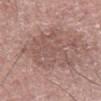Recorded during total-body skin imaging; not selected for excision or biopsy.
This is a white-light tile.
The lesion-visualizer software estimated a lesion area of about 24 mm², an eccentricity of roughly 0.85, and a symmetry-axis asymmetry near 0.35. The software also gave a border-irregularity rating of about 6/10, internal color variation of about 3 on a 0–10 scale, and peripheral color asymmetry of about 1.
The lesion's longest dimension is about 8.5 mm.
A lesion tile, about 15 mm wide, cut from a 3D total-body photograph.
Located on the leg.
The subject is a male aged 73 to 77.